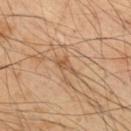biopsy status: total-body-photography surveillance lesion; no biopsy
size: ≈3 mm
patient: male, aged around 50
anatomic site: the upper back
tile lighting: cross-polarized
acquisition: 15 mm crop, total-body photography